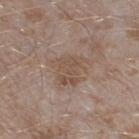The lesion was photographed on a routine skin check and not biopsied; there is no pathology result. This image is a 15 mm lesion crop taken from a total-body photograph. Automated image analysis of the tile measured an area of roughly 11 mm², an outline eccentricity of about 0.55 (0 = round, 1 = elongated), and two-axis asymmetry of about 0.35. The software also gave an average lesion color of about L≈51 a*≈15 b*≈25 (CIELAB), about 7 CIELAB-L* units darker than the surrounding skin, and a normalized lesion–skin contrast near 6. The software also gave border irregularity of about 5 on a 0–10 scale. The software also gave an automated nevus-likeness rating near 0 out of 100. Imaged with white-light lighting. A male patient, aged 43 to 47. On the leg.